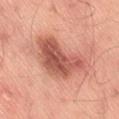Part of a total-body skin-imaging series; this lesion was reviewed on a skin check and was not flagged for biopsy.
Cropped from a whole-body photographic skin survey; the tile spans about 15 mm.
Imaged with white-light lighting.
A male patient, aged 48 to 52.
Approximately 7.5 mm at its widest.
On the lower back.